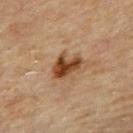biopsy_status: not biopsied; imaged during a skin examination
lighting: cross-polarized
site: upper back
lesion_size:
  long_diameter_mm_approx: 3.5
image:
  source: total-body photography crop
  field_of_view_mm: 15
patient:
  sex: male
  age_approx: 85+